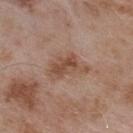imaging modality — ~15 mm tile from a whole-body skin photo
illumination — white-light illumination
location — the upper back
image-analysis metrics — a normalized lesion–skin contrast near 7; a border-irregularity rating of about 4.5/10, a color-variation rating of about 4.5/10, and radial color variation of about 1.5; a classifier nevus-likeness of about 0/100
patient — male, in their mid-50s